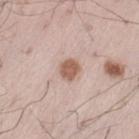No biopsy was performed on this lesion — it was imaged during a full skin examination and was not determined to be concerning. A male subject, roughly 55 years of age. Imaged with white-light lighting. Longest diameter approximately 2.5 mm. The lesion is located on the left thigh. A 15 mm close-up tile from a total-body photography series done for melanoma screening.